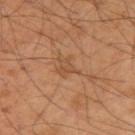follow-up = catalogued during a skin exam; not biopsied | imaging modality = 15 mm crop, total-body photography | patient = male, approximately 50 years of age | location = the left arm.